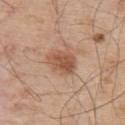Assessment:
The lesion was photographed on a routine skin check and not biopsied; there is no pathology result.
Image and clinical context:
A male patient approximately 55 years of age. From the upper back. This image is a 15 mm lesion crop taken from a total-body photograph. The lesion's longest dimension is about 3.5 mm.